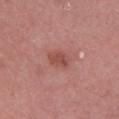The lesion was photographed on a routine skin check and not biopsied; there is no pathology result.
The tile uses white-light illumination.
Automated image analysis of the tile measured border irregularity of about 2.5 on a 0–10 scale and peripheral color asymmetry of about 1.
Cropped from a whole-body photographic skin survey; the tile spans about 15 mm.
The lesion's longest dimension is about 2.5 mm.
A male subject, aged 68 to 72.
Located on the right lower leg.